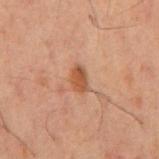| field | value |
|---|---|
| workup | catalogued during a skin exam; not biopsied |
| lighting | cross-polarized illumination |
| location | the mid back |
| subject | male, aged 58–62 |
| lesion size | ~2.5 mm (longest diameter) |
| image source | ~15 mm crop, total-body skin-cancer survey |